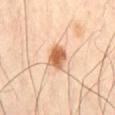| feature | finding |
|---|---|
| biopsy status | no biopsy performed (imaged during a skin exam) |